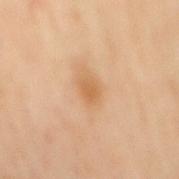Captured during whole-body skin photography for melanoma surveillance; the lesion was not biopsied.
A 15 mm close-up extracted from a 3D total-body photography capture.
Captured under cross-polarized illumination.
A male subject roughly 65 years of age.
Approximately 2.5 mm at its widest.
The total-body-photography lesion software estimated an automated nevus-likeness rating near 25 out of 100 and lesion-presence confidence of about 100/100.
From the back.